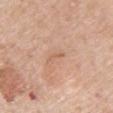Recorded during total-body skin imaging; not selected for excision or biopsy.